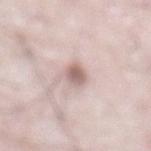Clinical impression:
Captured during whole-body skin photography for melanoma surveillance; the lesion was not biopsied.
Context:
A male patient in their mid- to late 70s. The tile uses white-light illumination. Automated tile analysis of the lesion measured a border-irregularity index near 2.5/10, a color-variation rating of about 3.5/10, and radial color variation of about 1. A close-up tile cropped from a whole-body skin photograph, about 15 mm across. Located on the abdomen.